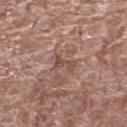Impression:
Recorded during total-body skin imaging; not selected for excision or biopsy.
Clinical summary:
A female subject in their mid- to late 70s. This is a white-light tile. Approximately 3 mm at its widest. A 15 mm close-up tile from a total-body photography series done for melanoma screening. From the left thigh.